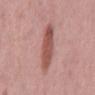Assessment: This lesion was catalogued during total-body skin photography and was not selected for biopsy. Background: The lesion is on the mid back. Captured under white-light illumination. Automated tile analysis of the lesion measured an area of roughly 12 mm² and a shape eccentricity near 0.9. The subject is a male about 60 years old. A close-up tile cropped from a whole-body skin photograph, about 15 mm across.